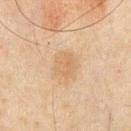follow-up: total-body-photography surveillance lesion; no biopsy | subject: male, in their mid- to late 40s | tile lighting: cross-polarized | image source: total-body-photography crop, ~15 mm field of view | site: the chest | lesion diameter: about 3 mm.